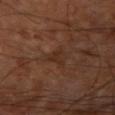Context:
A subject aged around 65. The tile uses cross-polarized illumination. Automated tile analysis of the lesion measured a lesion area of about 5 mm², an eccentricity of roughly 0.7, and two-axis asymmetry of about 0.5. It also reported an average lesion color of about L≈29 a*≈18 b*≈26 (CIELAB), roughly 5 lightness units darker than nearby skin, and a normalized lesion–skin contrast near 5. From the right upper arm. Measured at roughly 3.5 mm in maximum diameter. A roughly 15 mm field-of-view crop from a total-body skin photograph.